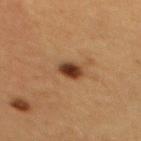workup=catalogued during a skin exam; not biopsied | body site=the mid back | subject=female, aged 38–42 | lesion diameter=about 2.5 mm | tile lighting=cross-polarized illumination | imaging modality=15 mm crop, total-body photography.